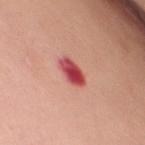Recorded during total-body skin imaging; not selected for excision or biopsy.
Automated tile analysis of the lesion measured a footprint of about 6 mm², an eccentricity of roughly 0.85, and a symmetry-axis asymmetry near 0.2. The analysis additionally found a lesion color around L≈50 a*≈40 b*≈26 in CIELAB, roughly 17 lightness units darker than nearby skin, and a normalized lesion–skin contrast near 11.5. It also reported a nevus-likeness score of about 0/100 and a detector confidence of about 100 out of 100 that the crop contains a lesion.
The lesion's longest dimension is about 3.5 mm.
The subject is a female aged 63 to 67.
Located on the front of the torso.
Captured under white-light illumination.
This image is a 15 mm lesion crop taken from a total-body photograph.